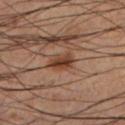notes = catalogued during a skin exam; not biopsied
site = the left lower leg
size = about 2.5 mm
acquisition = ~15 mm tile from a whole-body skin photo
patient = male, aged 58 to 62